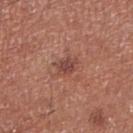Acquisition and patient details:
This is a white-light tile. On the right lower leg. A region of skin cropped from a whole-body photographic capture, roughly 15 mm wide. The lesion-visualizer software estimated border irregularity of about 2.5 on a 0–10 scale, a within-lesion color-variation index near 2.5/10, and peripheral color asymmetry of about 1. And it measured a classifier nevus-likeness of about 25/100 and lesion-presence confidence of about 100/100. The subject is a male aged approximately 70.